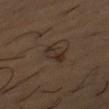Findings:
• illumination: cross-polarized
• diameter: ≈3.5 mm
• location: the left upper arm
• patient: male, approximately 55 years of age
• imaging modality: ~15 mm tile from a whole-body skin photo